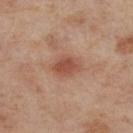Q: Is there a histopathology result?
A: imaged on a skin check; not biopsied
Q: Automated lesion metrics?
A: about 10 CIELAB-L* units darker than the surrounding skin and a normalized border contrast of about 7.5; border irregularity of about 1.5 on a 0–10 scale, a color-variation rating of about 3/10, and peripheral color asymmetry of about 1; a classifier nevus-likeness of about 85/100
Q: Who is the patient?
A: female, in their mid-50s
Q: What is the lesion's diameter?
A: about 3.5 mm
Q: Illumination type?
A: cross-polarized illumination
Q: What is the anatomic site?
A: the right lower leg
Q: How was this image acquired?
A: ~15 mm crop, total-body skin-cancer survey